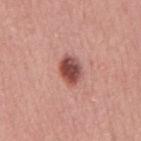Clinical summary: Longest diameter approximately 3.5 mm. A male patient, aged around 60. The total-body-photography lesion software estimated an outline eccentricity of about 0.7 (0 = round, 1 = elongated) and two-axis asymmetry of about 0.25. The analysis additionally found a lesion-to-skin contrast of about 11 (normalized; higher = more distinct). The tile uses white-light illumination. A close-up tile cropped from a whole-body skin photograph, about 15 mm across. From the mid back.